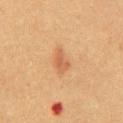follow-up — catalogued during a skin exam; not biopsied